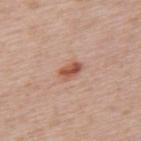Q: Was this lesion biopsied?
A: catalogued during a skin exam; not biopsied
Q: What are the patient's age and sex?
A: female, aged 58 to 62
Q: What is the anatomic site?
A: the upper back
Q: What kind of image is this?
A: total-body-photography crop, ~15 mm field of view